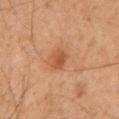– notes · catalogued during a skin exam; not biopsied